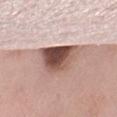image: ~15 mm tile from a whole-body skin photo; body site: the right thigh; subject: female, aged 53–57.